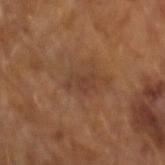Assessment:
Recorded during total-body skin imaging; not selected for excision or biopsy.
Clinical summary:
The subject is a male aged 63 to 67. A lesion tile, about 15 mm wide, cut from a 3D total-body photograph. The recorded lesion diameter is about 3.5 mm. An algorithmic analysis of the crop reported an area of roughly 4 mm², an outline eccentricity of about 0.85 (0 = round, 1 = elongated), and a symmetry-axis asymmetry near 0.45. Imaged with cross-polarized lighting.